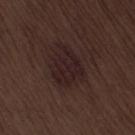{
  "biopsy_status": "not biopsied; imaged during a skin examination",
  "image": {
    "source": "total-body photography crop",
    "field_of_view_mm": 15
  },
  "site": "right thigh",
  "lighting": "white-light",
  "patient": {
    "sex": "male",
    "age_approx": 70
  },
  "automated_metrics": {
    "area_mm2_approx": 13.0,
    "eccentricity": 0.65,
    "shape_asymmetry": 0.3,
    "lesion_detection_confidence_0_100": 100
  }
}